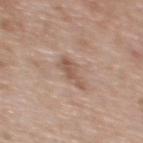Clinical impression: Imaged during a routine full-body skin examination; the lesion was not biopsied and no histopathology is available. Clinical summary: Imaged with white-light lighting. The subject is a female in their 40s. On the upper back. The total-body-photography lesion software estimated a footprint of about 4 mm², an outline eccentricity of about 0.9 (0 = round, 1 = elongated), and a shape-asymmetry score of about 0.5 (0 = symmetric). And it measured a mean CIELAB color near L≈54 a*≈18 b*≈27, a lesion–skin lightness drop of about 9, and a normalized lesion–skin contrast near 6.5. And it measured a border-irregularity rating of about 5.5/10, a within-lesion color-variation index near 1.5/10, and a peripheral color-asymmetry measure near 0.5. And it measured a detector confidence of about 100 out of 100 that the crop contains a lesion. Measured at roughly 3.5 mm in maximum diameter. A 15 mm close-up tile from a total-body photography series done for melanoma screening.